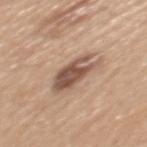The lesion was photographed on a routine skin check and not biopsied; there is no pathology result. A close-up tile cropped from a whole-body skin photograph, about 15 mm across. The lesion-visualizer software estimated a lesion area of about 11 mm², an outline eccentricity of about 0.8 (0 = round, 1 = elongated), and a shape-asymmetry score of about 0.2 (0 = symmetric). Approximately 5 mm at its widest. A male patient, aged around 50. On the mid back. This is a white-light tile.